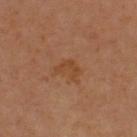Image and clinical context:
Automated tile analysis of the lesion measured an average lesion color of about L≈43 a*≈22 b*≈35 (CIELAB), about 6 CIELAB-L* units darker than the surrounding skin, and a lesion-to-skin contrast of about 6 (normalized; higher = more distinct). The software also gave a classifier nevus-likeness of about 0/100 and a detector confidence of about 100 out of 100 that the crop contains a lesion. A 15 mm close-up extracted from a 3D total-body photography capture. This is a cross-polarized tile. Longest diameter approximately 2.5 mm. A female subject in their mid-40s. On the upper back.